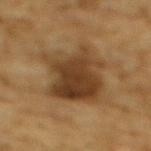notes=catalogued during a skin exam; not biopsied | subject=male, aged around 85 or older | site=the upper back | tile lighting=cross-polarized illumination | imaging modality=~15 mm tile from a whole-body skin photo | automated metrics=an area of roughly 28 mm², an eccentricity of roughly 0.65, and a symmetry-axis asymmetry near 0.25; a border-irregularity index near 3.5/10 and radial color variation of about 2; an automated nevus-likeness rating near 50 out of 100.